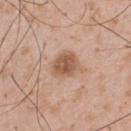The lesion is located on the upper back. Imaged with white-light lighting. Cropped from a total-body skin-imaging series; the visible field is about 15 mm. A male subject aged 53 to 57.